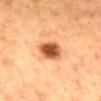The lesion was photographed on a routine skin check and not biopsied; there is no pathology result.
About 3.5 mm across.
The lesion-visualizer software estimated a lesion area of about 8 mm², an eccentricity of roughly 0.5, and a symmetry-axis asymmetry near 0.25. It also reported border irregularity of about 2 on a 0–10 scale, a color-variation rating of about 5/10, and radial color variation of about 1.5.
A 15 mm close-up tile from a total-body photography series done for melanoma screening.
Captured under cross-polarized illumination.
A female patient, roughly 60 years of age.
Located on the mid back.